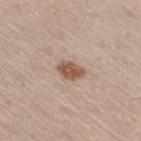Imaged during a routine full-body skin examination; the lesion was not biopsied and no histopathology is available.
Measured at roughly 3 mm in maximum diameter.
The subject is a male aged approximately 60.
Captured under white-light illumination.
The lesion is located on the right thigh.
An algorithmic analysis of the crop reported a footprint of about 5 mm² and a shape-asymmetry score of about 0.25 (0 = symmetric). And it measured a border-irregularity index near 2.5/10, a within-lesion color-variation index near 2.5/10, and a peripheral color-asymmetry measure near 1. And it measured a nevus-likeness score of about 95/100 and a detector confidence of about 100 out of 100 that the crop contains a lesion.
A 15 mm close-up extracted from a 3D total-body photography capture.